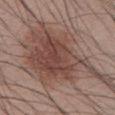biopsy_status: not biopsied; imaged during a skin examination
lighting: white-light
patient:
  sex: male
  age_approx: 40
site: abdomen
image:
  source: total-body photography crop
  field_of_view_mm: 15
automated_metrics:
  cielab_L: 46
  cielab_a: 18
  cielab_b: 23
  vs_skin_darker_L: 11.0
  vs_skin_contrast_norm: 8.0